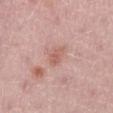Q: What is the imaging modality?
A: 15 mm crop, total-body photography
Q: What lighting was used for the tile?
A: white-light illumination
Q: Patient demographics?
A: female, about 50 years old
Q: Lesion location?
A: the leg